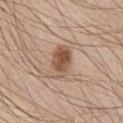Imaged during a routine full-body skin examination; the lesion was not biopsied and no histopathology is available. Cropped from a total-body skin-imaging series; the visible field is about 15 mm. An algorithmic analysis of the crop reported a mean CIELAB color near L≈54 a*≈18 b*≈29 and a lesion-to-skin contrast of about 8.5 (normalized; higher = more distinct). A male subject, aged 43 to 47. From the chest. About 4 mm across.